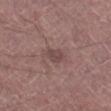notes = catalogued during a skin exam; not biopsied | image source = ~15 mm tile from a whole-body skin photo | lesion size = about 2.5 mm | image-analysis metrics = an area of roughly 4 mm² and an eccentricity of roughly 0.8 | site = the left lower leg | patient = male, aged 43 to 47 | illumination = white-light illumination.